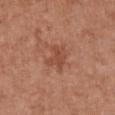Assessment: Recorded during total-body skin imaging; not selected for excision or biopsy. Background: Cropped from a total-body skin-imaging series; the visible field is about 15 mm. On the chest. A female patient, in their mid- to late 60s.